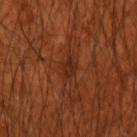This lesion was catalogued during total-body skin photography and was not selected for biopsy. The tile uses cross-polarized illumination. Automated image analysis of the tile measured a mean CIELAB color near L≈27 a*≈24 b*≈30, a lesion–skin lightness drop of about 6, and a lesion-to-skin contrast of about 7 (normalized; higher = more distinct). The software also gave a border-irregularity rating of about 2.5/10 and a peripheral color-asymmetry measure near 1. A male patient, about 70 years old. Measured at roughly 3 mm in maximum diameter. On the right upper arm. A roughly 15 mm field-of-view crop from a total-body skin photograph.